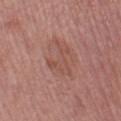Notes:
– workup: total-body-photography surveillance lesion; no biopsy
– imaging modality: total-body-photography crop, ~15 mm field of view
– size: about 4 mm
– subject: female, aged 48 to 52
– body site: the left thigh
– automated metrics: an area of roughly 7 mm² and a shape eccentricity near 0.75; a border-irregularity rating of about 9/10, internal color variation of about 2.5 on a 0–10 scale, and peripheral color asymmetry of about 1; a nevus-likeness score of about 0/100 and a detector confidence of about 100 out of 100 that the crop contains a lesion